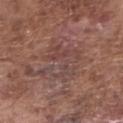Captured during whole-body skin photography for melanoma surveillance; the lesion was not biopsied. The recorded lesion diameter is about 6 mm. A 15 mm crop from a total-body photograph taken for skin-cancer surveillance. A male patient, approximately 75 years of age. On the right forearm. The total-body-photography lesion software estimated a footprint of about 18 mm², a shape eccentricity near 0.7, and a shape-asymmetry score of about 0.4 (0 = symmetric). It also reported roughly 5 lightness units darker than nearby skin and a normalized border contrast of about 5.